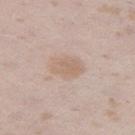The lesion was photographed on a routine skin check and not biopsied; there is no pathology result. Located on the left thigh. A female patient about 25 years old. The lesion-visualizer software estimated a lesion area of about 7 mm² and an outline eccentricity of about 0.75 (0 = round, 1 = elongated). And it measured a classifier nevus-likeness of about 5/100. Imaged with white-light lighting. The lesion's longest dimension is about 3.5 mm. A 15 mm crop from a total-body photograph taken for skin-cancer surveillance.